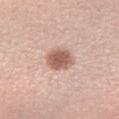No biopsy was performed on this lesion — it was imaged during a full skin examination and was not determined to be concerning.
A region of skin cropped from a whole-body photographic capture, roughly 15 mm wide.
This is a white-light tile.
Automated image analysis of the tile measured a mean CIELAB color near L≈59 a*≈21 b*≈28, about 14 CIELAB-L* units darker than the surrounding skin, and a normalized border contrast of about 9. The software also gave an automated nevus-likeness rating near 95 out of 100.
The lesion's longest dimension is about 3 mm.
Located on the left forearm.
A female subject, aged around 25.